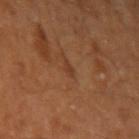Case summary:
• workup — total-body-photography surveillance lesion; no biopsy
• patient — male, in their 60s
• size — about 1.5 mm
• location — the left upper arm
• image source — total-body-photography crop, ~15 mm field of view
• lighting — cross-polarized
• automated metrics — a lesion area of about 1.5 mm², a shape eccentricity near 0.8, and two-axis asymmetry of about 0.45; a lesion color around L≈34 a*≈20 b*≈29 in CIELAB, roughly 6 lightness units darker than nearby skin, and a normalized border contrast of about 5.5; a border-irregularity rating of about 3.5/10 and radial color variation of about 0